A 15 mm crop from a total-body photograph taken for skin-cancer surveillance. On the right upper arm. The tile uses white-light illumination. A female subject, aged around 40. About 5.5 mm across — 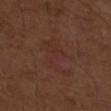histopathology=a compound melanocytic nevus.